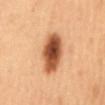notes: no biopsy performed (imaged during a skin exam); acquisition: ~15 mm tile from a whole-body skin photo; body site: the mid back; subject: female, aged 58 to 62.